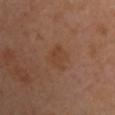Q: Was this lesion biopsied?
A: catalogued during a skin exam; not biopsied
Q: How was this image acquired?
A: ~15 mm tile from a whole-body skin photo
Q: Lesion size?
A: ~3 mm (longest diameter)
Q: How was the tile lit?
A: cross-polarized illumination
Q: Patient demographics?
A: female, in their 60s
Q: Where on the body is the lesion?
A: the chest